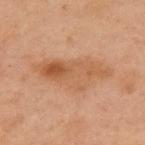Notes:
- workup · catalogued during a skin exam; not biopsied
- body site · the left arm
- image · ~15 mm tile from a whole-body skin photo
- illumination · cross-polarized illumination
- patient · female, in their mid-50s